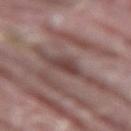Assessment: The lesion was photographed on a routine skin check and not biopsied; there is no pathology result. Clinical summary: From the leg. This is a white-light tile. Cropped from a total-body skin-imaging series; the visible field is about 15 mm. A male patient, aged 38 to 42.